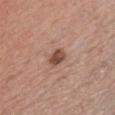Clinical impression:
No biopsy was performed on this lesion — it was imaged during a full skin examination and was not determined to be concerning.
Image and clinical context:
A 15 mm close-up tile from a total-body photography series done for melanoma screening. Captured under white-light illumination. The subject is a male aged approximately 40. From the chest. Approximately 2.5 mm at its widest.